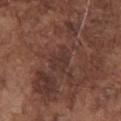notes: catalogued during a skin exam; not biopsied.